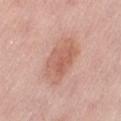notes=imaged on a skin check; not biopsied
subject=female, in their 50s
size=about 5.5 mm
tile lighting=white-light
image=15 mm crop, total-body photography
anatomic site=the abdomen